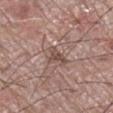Q: Was this lesion biopsied?
A: catalogued during a skin exam; not biopsied
Q: Patient demographics?
A: male, in their 70s
Q: What did automated image analysis measure?
A: a lesion area of about 3.5 mm², an eccentricity of roughly 0.85, and a symmetry-axis asymmetry near 0.55
Q: What is the anatomic site?
A: the leg
Q: What is the imaging modality?
A: 15 mm crop, total-body photography